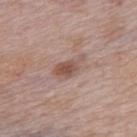This is a white-light tile. Located on the upper back. The recorded lesion diameter is about 4 mm. Cropped from a whole-body photographic skin survey; the tile spans about 15 mm. A female subject, in their mid-60s.